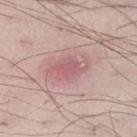| key | value |
|---|---|
| biopsy status | no biopsy performed (imaged during a skin exam) |
| location | the left thigh |
| imaging modality | ~15 mm crop, total-body skin-cancer survey |
| subject | male, aged 33 to 37 |